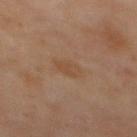Q: Patient demographics?
A: female, aged around 50
Q: Illumination type?
A: cross-polarized
Q: Lesion location?
A: the mid back
Q: What is the imaging modality?
A: ~15 mm tile from a whole-body skin photo